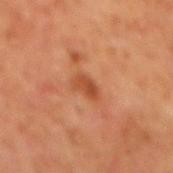Findings:
– notes — total-body-photography surveillance lesion; no biopsy
– image source — total-body-photography crop, ~15 mm field of view
– subject — male, roughly 60 years of age
– TBP lesion metrics — a footprint of about 4 mm² and an eccentricity of roughly 0.85; an average lesion color of about L≈44 a*≈27 b*≈35 (CIELAB) and a lesion–skin lightness drop of about 9; an automated nevus-likeness rating near 15 out of 100 and a detector confidence of about 100 out of 100 that the crop contains a lesion
– anatomic site — the chest
– diameter — ~3 mm (longest diameter)
– lighting — cross-polarized illumination